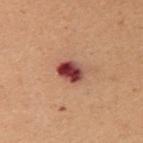Impression: The lesion was tiled from a total-body skin photograph and was not biopsied. Image and clinical context: This is a cross-polarized tile. About 3 mm across. The patient is a female aged 48 to 52. Cropped from a total-body skin-imaging series; the visible field is about 15 mm. The lesion-visualizer software estimated a footprint of about 6 mm² and a shape eccentricity near 0.6. The software also gave a classifier nevus-likeness of about 0/100 and a detector confidence of about 100 out of 100 that the crop contains a lesion. On the chest.